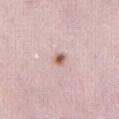follow-up: catalogued during a skin exam; not biopsied | size: ~1.5 mm (longest diameter) | body site: the abdomen | subject: female, approximately 30 years of age | acquisition: ~15 mm tile from a whole-body skin photo | lighting: white-light illumination | TBP lesion metrics: about 14 CIELAB-L* units darker than the surrounding skin and a lesion-to-skin contrast of about 10 (normalized; higher = more distinct); a color-variation rating of about 2.5/10 and radial color variation of about 1; a nevus-likeness score of about 95/100 and a lesion-detection confidence of about 100/100.